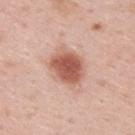Findings:
• workup · imaged on a skin check; not biopsied
• site · the upper back
• subject · male, roughly 35 years of age
• automated lesion analysis · a lesion area of about 14 mm², an eccentricity of roughly 0.7, and a symmetry-axis asymmetry near 0.15; an average lesion color of about L≈58 a*≈24 b*≈29 (CIELAB), a lesion–skin lightness drop of about 14, and a normalized border contrast of about 9.5; an automated nevus-likeness rating near 100 out of 100 and a lesion-detection confidence of about 100/100
• diameter · ≈5 mm
• image · ~15 mm tile from a whole-body skin photo